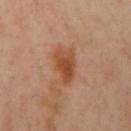Assessment:
The lesion was photographed on a routine skin check and not biopsied; there is no pathology result.
Background:
A female subject aged 38–42. Automated tile analysis of the lesion measured a within-lesion color-variation index near 4/10 and a peripheral color-asymmetry measure near 1. The software also gave an automated nevus-likeness rating near 80 out of 100 and a lesion-detection confidence of about 100/100. From the left arm. Captured under cross-polarized illumination. Measured at roughly 4 mm in maximum diameter. Cropped from a whole-body photographic skin survey; the tile spans about 15 mm.